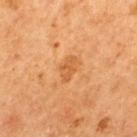biopsy_status: not biopsied; imaged during a skin examination
lesion_size:
  long_diameter_mm_approx: 3.0
image:
  source: total-body photography crop
  field_of_view_mm: 15
site: upper back
automated_metrics:
  area_mm2_approx: 5.0
  eccentricity: 0.85
  shape_asymmetry: 0.3
  border_irregularity_0_10: 3.0
  color_variation_0_10: 2.0
  peripheral_color_asymmetry: 0.5
  nevus_likeness_0_100: 30
  lesion_detection_confidence_0_100: 100
patient:
  sex: male
  age_approx: 65
lighting: cross-polarized